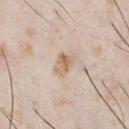This lesion was catalogued during total-body skin photography and was not selected for biopsy. A male patient, in their mid- to late 20s. A region of skin cropped from a whole-body photographic capture, roughly 15 mm wide. On the front of the torso. Automated image analysis of the tile measured an area of roughly 4.5 mm², a shape eccentricity near 0.7, and two-axis asymmetry of about 0.3. And it measured a mean CIELAB color near L≈65 a*≈16 b*≈31, roughly 10 lightness units darker than nearby skin, and a lesion-to-skin contrast of about 7.5 (normalized; higher = more distinct). The analysis additionally found border irregularity of about 2.5 on a 0–10 scale, a within-lesion color-variation index near 5.5/10, and radial color variation of about 2.